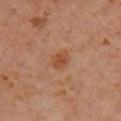Impression:
The lesion was photographed on a routine skin check and not biopsied; there is no pathology result.
Image and clinical context:
The subject is a male aged approximately 60. On the leg. A lesion tile, about 15 mm wide, cut from a 3D total-body photograph.